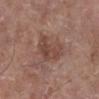Background:
The subject is a female about 75 years old. This image is a 15 mm lesion crop taken from a total-body photograph. Captured under white-light illumination. The lesion is on the right lower leg. Measured at roughly 4.5 mm in maximum diameter.